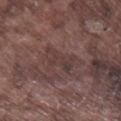biopsy status: total-body-photography surveillance lesion; no biopsy | imaging modality: ~15 mm tile from a whole-body skin photo | location: the right lower leg | subject: male, roughly 75 years of age.